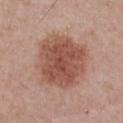* notes · imaged on a skin check; not biopsied
* automated lesion analysis · an eccentricity of roughly 0.4 and two-axis asymmetry of about 0.1; a mean CIELAB color near L≈52 a*≈23 b*≈27, a lesion–skin lightness drop of about 12, and a normalized border contrast of about 8.5; a nevus-likeness score of about 85/100 and a lesion-detection confidence of about 100/100
* acquisition · ~15 mm tile from a whole-body skin photo
* lighting · white-light
* patient · male, approximately 55 years of age
* anatomic site · the chest